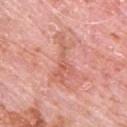Findings:
– biopsy status: imaged on a skin check; not biopsied
– site: the upper back
– subject: male, aged 78 to 82
– diameter: about 6 mm
– imaging modality: ~15 mm tile from a whole-body skin photo
– automated metrics: an area of roughly 9 mm² and a symmetry-axis asymmetry near 0.7; a border-irregularity rating of about 8.5/10 and a peripheral color-asymmetry measure near 1